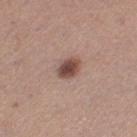{"biopsy_status": "not biopsied; imaged during a skin examination", "site": "left thigh", "lighting": "white-light", "automated_metrics": {"cielab_L": 47, "cielab_a": 20, "cielab_b": 24, "vs_skin_darker_L": 15.0, "vs_skin_contrast_norm": 10.5, "nevus_likeness_0_100": 95, "lesion_detection_confidence_0_100": 100}, "patient": {"sex": "female", "age_approx": 30}, "image": {"source": "total-body photography crop", "field_of_view_mm": 15}, "lesion_size": {"long_diameter_mm_approx": 3.0}}